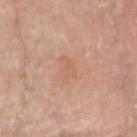workup=no biopsy performed (imaged during a skin exam); site=the head or neck; subject=male, aged approximately 80; image source=~15 mm crop, total-body skin-cancer survey; lesion diameter=about 3 mm; lighting=white-light.